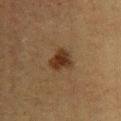Impression:
Part of a total-body skin-imaging series; this lesion was reviewed on a skin check and was not flagged for biopsy.
Image and clinical context:
A male subject, roughly 75 years of age. Automated tile analysis of the lesion measured an area of roughly 6 mm², a shape eccentricity near 0.65, and two-axis asymmetry of about 0.25. The software also gave roughly 10 lightness units darker than nearby skin and a lesion-to-skin contrast of about 10.5 (normalized; higher = more distinct). And it measured border irregularity of about 2.5 on a 0–10 scale, internal color variation of about 3 on a 0–10 scale, and peripheral color asymmetry of about 1. It also reported an automated nevus-likeness rating near 90 out of 100 and a lesion-detection confidence of about 100/100. Cropped from a whole-body photographic skin survey; the tile spans about 15 mm. About 3 mm across. Located on the left lower leg.